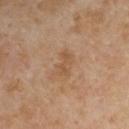Part of a total-body skin-imaging series; this lesion was reviewed on a skin check and was not flagged for biopsy. Automated image analysis of the tile measured a lesion color around L≈52 a*≈19 b*≈34 in CIELAB and a lesion–skin lightness drop of about 7. The software also gave an automated nevus-likeness rating near 0 out of 100. A male patient, aged approximately 55. Cropped from a total-body skin-imaging series; the visible field is about 15 mm. Captured under cross-polarized illumination. Longest diameter approximately 3 mm. On the left upper arm.